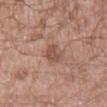{"lighting": "white-light", "image": {"source": "total-body photography crop", "field_of_view_mm": 15}, "automated_metrics": {"vs_skin_darker_L": 10.0, "vs_skin_contrast_norm": 7.0, "nevus_likeness_0_100": 5, "lesion_detection_confidence_0_100": 100}, "lesion_size": {"long_diameter_mm_approx": 3.0}, "site": "mid back", "patient": {"sex": "male", "age_approx": 55}}